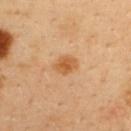follow-up: total-body-photography surveillance lesion; no biopsy | illumination: cross-polarized | automated lesion analysis: an area of roughly 5 mm², an outline eccentricity of about 0.7 (0 = round, 1 = elongated), and a symmetry-axis asymmetry near 0.15; a lesion color around L≈58 a*≈23 b*≈43 in CIELAB, roughly 10 lightness units darker than nearby skin, and a lesion-to-skin contrast of about 8 (normalized; higher = more distinct); border irregularity of about 1.5 on a 0–10 scale and peripheral color asymmetry of about 1; a detector confidence of about 100 out of 100 that the crop contains a lesion | subject: male, aged approximately 40 | imaging modality: ~15 mm crop, total-body skin-cancer survey | anatomic site: the upper back.